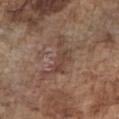Clinical impression: No biopsy was performed on this lesion — it was imaged during a full skin examination and was not determined to be concerning. Acquisition and patient details: A 15 mm close-up tile from a total-body photography series done for melanoma screening. The subject is a male aged around 75. From the chest. The lesion-visualizer software estimated border irregularity of about 9 on a 0–10 scale and a within-lesion color-variation index near 3.5/10. The software also gave a lesion-detection confidence of about 85/100.